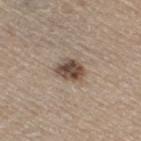Findings:
• workup — catalogued during a skin exam; not biopsied
• imaging modality — ~15 mm crop, total-body skin-cancer survey
• patient — female, about 70 years old
• size — ~3.5 mm (longest diameter)
• anatomic site — the right thigh